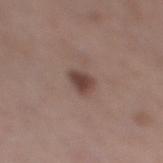| feature | finding |
|---|---|
| patient | female, aged 38–42 |
| automated lesion analysis | a lesion area of about 4.5 mm², an outline eccentricity of about 0.55 (0 = round, 1 = elongated), and a shape-asymmetry score of about 0.3 (0 = symmetric); an average lesion color of about L≈43 a*≈17 b*≈22 (CIELAB), about 11 CIELAB-L* units darker than the surrounding skin, and a lesion-to-skin contrast of about 9 (normalized; higher = more distinct) |
| lighting | white-light illumination |
| imaging modality | 15 mm crop, total-body photography |
| body site | the right lower leg |